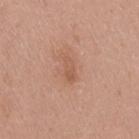Part of a total-body skin-imaging series; this lesion was reviewed on a skin check and was not flagged for biopsy. Approximately 2.5 mm at its widest. Cropped from a whole-body photographic skin survey; the tile spans about 15 mm. A female patient, approximately 40 years of age. Located on the leg. Automated tile analysis of the lesion measured roughly 7 lightness units darker than nearby skin and a normalized lesion–skin contrast near 5.5. The analysis additionally found border irregularity of about 3 on a 0–10 scale, a color-variation rating of about 0.5/10, and radial color variation of about 0.